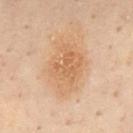| key | value |
|---|---|
| workup | imaged on a skin check; not biopsied |
| lighting | cross-polarized |
| acquisition | total-body-photography crop, ~15 mm field of view |
| diameter | ≈6 mm |
| patient | male, in their 50s |
| location | the mid back |
| TBP lesion metrics | an area of roughly 20 mm², an outline eccentricity of about 0.65 (0 = round, 1 = elongated), and a shape-asymmetry score of about 0.2 (0 = symmetric); a mean CIELAB color near L≈52 a*≈16 b*≈31 and a lesion-to-skin contrast of about 6 (normalized; higher = more distinct); a border-irregularity rating of about 2.5/10, internal color variation of about 3 on a 0–10 scale, and radial color variation of about 1; an automated nevus-likeness rating near 25 out of 100 and a detector confidence of about 100 out of 100 that the crop contains a lesion |